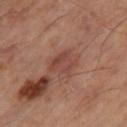Assessment: Recorded during total-body skin imaging; not selected for excision or biopsy.